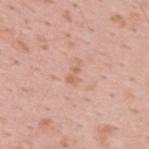Q: Is there a histopathology result?
A: catalogued during a skin exam; not biopsied
Q: What lighting was used for the tile?
A: white-light illumination
Q: What is the imaging modality?
A: ~15 mm crop, total-body skin-cancer survey
Q: What is the anatomic site?
A: the back
Q: What did automated image analysis measure?
A: a lesion area of about 2.5 mm², a shape eccentricity near 0.9, and a shape-asymmetry score of about 0.45 (0 = symmetric); a border-irregularity index near 5.5/10, internal color variation of about 0 on a 0–10 scale, and a peripheral color-asymmetry measure near 0; an automated nevus-likeness rating near 0 out of 100 and lesion-presence confidence of about 100/100
Q: Patient demographics?
A: male, about 35 years old
Q: What is the lesion's diameter?
A: ≈2.5 mm